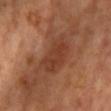The lesion was tiled from a total-body skin photograph and was not biopsied. Imaged with cross-polarized lighting. The patient is a female aged 63 to 67. From the chest. This image is a 15 mm lesion crop taken from a total-body photograph. The recorded lesion diameter is about 6 mm.